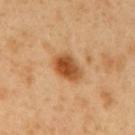Captured during whole-body skin photography for melanoma surveillance; the lesion was not biopsied. A male subject in their 50s. A lesion tile, about 15 mm wide, cut from a 3D total-body photograph. Imaged with cross-polarized lighting. The recorded lesion diameter is about 4 mm. Automated tile analysis of the lesion measured a lesion area of about 8.5 mm², an eccentricity of roughly 0.75, and a symmetry-axis asymmetry near 0.25. The software also gave a mean CIELAB color near L≈51 a*≈24 b*≈41, about 14 CIELAB-L* units darker than the surrounding skin, and a lesion-to-skin contrast of about 10 (normalized; higher = more distinct). And it measured border irregularity of about 2 on a 0–10 scale, a within-lesion color-variation index near 6/10, and peripheral color asymmetry of about 2. On the mid back.